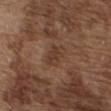The lesion was photographed on a routine skin check and not biopsied; there is no pathology result. A close-up tile cropped from a whole-body skin photograph, about 15 mm across. From the front of the torso. Longest diameter approximately 3.5 mm. Automated tile analysis of the lesion measured a lesion area of about 6 mm², an outline eccentricity of about 0.85 (0 = round, 1 = elongated), and a symmetry-axis asymmetry near 0.25. The software also gave a border-irregularity rating of about 3/10, a color-variation rating of about 2/10, and peripheral color asymmetry of about 0.5. The analysis additionally found a lesion-detection confidence of about 100/100. Imaged with white-light lighting. A male subject aged 73–77.